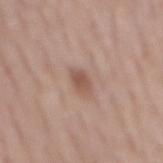This lesion was catalogued during total-body skin photography and was not selected for biopsy.
The subject is a male aged around 60.
The lesion is on the mid back.
A 15 mm crop from a total-body photograph taken for skin-cancer surveillance.
Longest diameter approximately 2.5 mm.